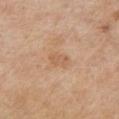No biopsy was performed on this lesion — it was imaged during a full skin examination and was not determined to be concerning.
This image is a 15 mm lesion crop taken from a total-body photograph.
Approximately 3 mm at its widest.
A male patient, aged 63 to 67.
On the chest.
Captured under white-light illumination.